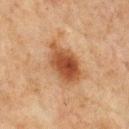Impression:
The lesion was photographed on a routine skin check and not biopsied; there is no pathology result.
Clinical summary:
Approximately 5.5 mm at its widest. A 15 mm crop from a total-body photograph taken for skin-cancer surveillance. The lesion is located on the chest. Captured under cross-polarized illumination. A male patient, in their mid- to late 70s.